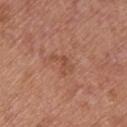{
  "biopsy_status": "not biopsied; imaged during a skin examination",
  "image": {
    "source": "total-body photography crop",
    "field_of_view_mm": 15
  },
  "lighting": "white-light",
  "site": "back",
  "lesion_size": {
    "long_diameter_mm_approx": 3.0
  },
  "patient": {
    "sex": "male",
    "age_approx": 55
  }
}